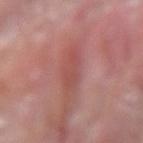The lesion was tiled from a total-body skin photograph and was not biopsied.
From the right forearm.
This is a white-light tile.
Automated tile analysis of the lesion measured an average lesion color of about L≈51 a*≈28 b*≈25 (CIELAB), roughly 7 lightness units darker than nearby skin, and a normalized lesion–skin contrast near 5. The software also gave border irregularity of about 2.5 on a 0–10 scale, a color-variation rating of about 2/10, and radial color variation of about 0.5.
The patient is a male aged 73–77.
The recorded lesion diameter is about 4.5 mm.
A region of skin cropped from a whole-body photographic capture, roughly 15 mm wide.